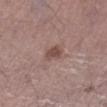• follow-up · imaged on a skin check; not biopsied
• automated lesion analysis · an area of roughly 4 mm² and a shape-asymmetry score of about 0.25 (0 = symmetric); internal color variation of about 1.5 on a 0–10 scale; a classifier nevus-likeness of about 55/100
• anatomic site · the right lower leg
• patient · male, about 55 years old
• image source · ~15 mm crop, total-body skin-cancer survey